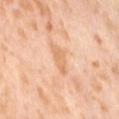The lesion was photographed on a routine skin check and not biopsied; there is no pathology result.
Cropped from a total-body skin-imaging series; the visible field is about 15 mm.
The total-body-photography lesion software estimated a footprint of about 4.5 mm², a shape eccentricity near 0.9, and a symmetry-axis asymmetry near 0.3. And it measured a lesion–skin lightness drop of about 8 and a lesion-to-skin contrast of about 5.5 (normalized; higher = more distinct).
About 3.5 mm across.
A female patient, aged 53–57.
On the lower back.